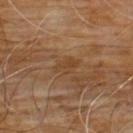{
  "biopsy_status": "not biopsied; imaged during a skin examination",
  "image": {
    "source": "total-body photography crop",
    "field_of_view_mm": 15
  },
  "patient": {
    "sex": "male",
    "age_approx": 60
  },
  "lesion_size": {
    "long_diameter_mm_approx": 3.0
  },
  "site": "chest",
  "automated_metrics": {
    "area_mm2_approx": 4.0,
    "eccentricity": 0.8,
    "shape_asymmetry": 0.25,
    "cielab_L": 40,
    "cielab_a": 18,
    "cielab_b": 33,
    "vs_skin_darker_L": 6.0,
    "vs_skin_contrast_norm": 5.5,
    "nevus_likeness_0_100": 0,
    "lesion_detection_confidence_0_100": 100
  },
  "lighting": "cross-polarized"
}